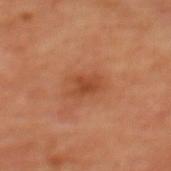Clinical impression: Part of a total-body skin-imaging series; this lesion was reviewed on a skin check and was not flagged for biopsy.